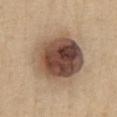Clinical impression: Recorded during total-body skin imaging; not selected for excision or biopsy. Context: The patient is a female aged 58–62. Captured under white-light illumination. From the chest. A 15 mm close-up tile from a total-body photography series done for melanoma screening.